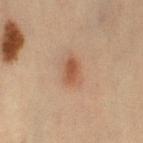biopsy_status: not biopsied; imaged during a skin examination
patient:
  sex: female
  age_approx: 45
automated_metrics:
  area_mm2_approx: 5.5
  eccentricity: 0.8
  shape_asymmetry: 0.15
  cielab_L: 46
  cielab_a: 20
  cielab_b: 29
  vs_skin_darker_L: 9.0
  vs_skin_contrast_norm: 7.0
  border_irregularity_0_10: 1.5
  color_variation_0_10: 3.0
  peripheral_color_asymmetry: 1.0
  lesion_detection_confidence_0_100: 100
site: left thigh
image:
  source: total-body photography crop
  field_of_view_mm: 15
lighting: cross-polarized